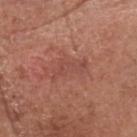Findings:
• notes · no biopsy performed (imaged during a skin exam)
• tile lighting · white-light illumination
• diameter · about 4.5 mm
• automated lesion analysis · an area of roughly 5 mm², a shape eccentricity near 0.9, and a symmetry-axis asymmetry near 0.55; a lesion color around L≈48 a*≈26 b*≈26 in CIELAB and a lesion-to-skin contrast of about 5 (normalized; higher = more distinct); a classifier nevus-likeness of about 0/100 and lesion-presence confidence of about 100/100
• subject · male, in their mid-70s
• acquisition · 15 mm crop, total-body photography
• location · the head or neck